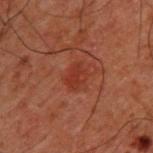<case>
<biopsy_status>not biopsied; imaged during a skin examination</biopsy_status>
<lesion_size>
  <long_diameter_mm_approx>2.5</long_diameter_mm_approx>
</lesion_size>
<lighting>cross-polarized</lighting>
<patient>
  <sex>male</sex>
  <age_approx>50</age_approx>
</patient>
<site>upper back</site>
<image>
  <source>total-body photography crop</source>
  <field_of_view_mm>15</field_of_view_mm>
</image>
</case>